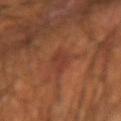Q: Was this lesion biopsied?
A: total-body-photography surveillance lesion; no biopsy
Q: How was this image acquired?
A: ~15 mm crop, total-body skin-cancer survey
Q: How large is the lesion?
A: about 3.5 mm
Q: What is the anatomic site?
A: the left forearm
Q: Patient demographics?
A: male, aged 63 to 67
Q: What lighting was used for the tile?
A: cross-polarized